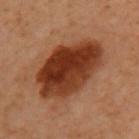notes: imaged on a skin check; not biopsied
acquisition: ~15 mm crop, total-body skin-cancer survey
anatomic site: the left arm
patient: female, approximately 60 years of age
illumination: cross-polarized